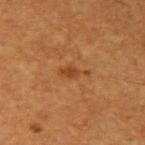Clinical impression: The lesion was photographed on a routine skin check and not biopsied; there is no pathology result. Background: A 15 mm close-up extracted from a 3D total-body photography capture. From the right upper arm. The patient is a male in their 60s.